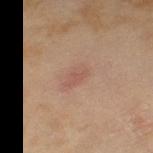Clinical impression:
Recorded during total-body skin imaging; not selected for excision or biopsy.
Image and clinical context:
A 15 mm close-up tile from a total-body photography series done for melanoma screening. The recorded lesion diameter is about 3 mm. A female patient aged 58 to 62. From the left thigh. This is a cross-polarized tile.